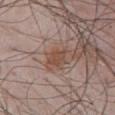  biopsy_status: not biopsied; imaged during a skin examination
  lighting: white-light
  image:
    source: total-body photography crop
    field_of_view_mm: 15
  automated_metrics:
    vs_skin_darker_L: 8.0
    vs_skin_contrast_norm: 7.0
    border_irregularity_0_10: 3.5
    color_variation_0_10: 2.0
    peripheral_color_asymmetry: 1.0
  site: chest
  lesion_size:
    long_diameter_mm_approx: 3.5
  patient:
    sex: male
    age_approx: 55